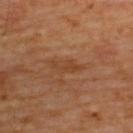| field | value |
|---|---|
| workup | total-body-photography surveillance lesion; no biopsy |
| acquisition | ~15 mm tile from a whole-body skin photo |
| body site | the upper back |
| subject | male, in their 60s |
| lesion size | ~3.5 mm (longest diameter) |
| automated metrics | an average lesion color of about L≈41 a*≈22 b*≈33 (CIELAB) and a normalized border contrast of about 5.5 |